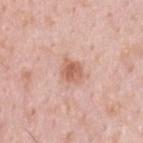Impression: Captured during whole-body skin photography for melanoma surveillance; the lesion was not biopsied. Clinical summary: From the upper back. A male subject, aged around 35. A roughly 15 mm field-of-view crop from a total-body skin photograph. This is a white-light tile.